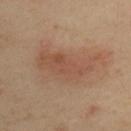The lesion was photographed on a routine skin check and not biopsied; there is no pathology result.
About 7 mm across.
The tile uses cross-polarized illumination.
A female patient, in their 40s.
From the left upper arm.
Cropped from a total-body skin-imaging series; the visible field is about 15 mm.